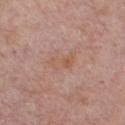Assessment:
No biopsy was performed on this lesion — it was imaged during a full skin examination and was not determined to be concerning.
Image and clinical context:
Cropped from a whole-body photographic skin survey; the tile spans about 15 mm. The recorded lesion diameter is about 3.5 mm. The tile uses white-light illumination. From the chest. The subject is a male aged 73–77.